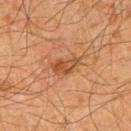Q: Was this lesion biopsied?
A: total-body-photography surveillance lesion; no biopsy
Q: What are the patient's age and sex?
A: male, roughly 65 years of age
Q: Automated lesion metrics?
A: a mean CIELAB color near L≈42 a*≈21 b*≈32, about 8 CIELAB-L* units darker than the surrounding skin, and a lesion-to-skin contrast of about 7 (normalized; higher = more distinct); a peripheral color-asymmetry measure near 1; an automated nevus-likeness rating near 55 out of 100 and a detector confidence of about 100 out of 100 that the crop contains a lesion
Q: How large is the lesion?
A: ~3.5 mm (longest diameter)
Q: Illumination type?
A: cross-polarized
Q: Where on the body is the lesion?
A: the arm
Q: What is the imaging modality?
A: ~15 mm crop, total-body skin-cancer survey